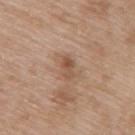Assessment:
The lesion was tiled from a total-body skin photograph and was not biopsied.
Clinical summary:
A female subject aged 73–77. Automated tile analysis of the lesion measured a footprint of about 3.5 mm², an outline eccentricity of about 0.8 (0 = round, 1 = elongated), and a shape-asymmetry score of about 0.35 (0 = symmetric). The analysis additionally found an average lesion color of about L≈52 a*≈19 b*≈30 (CIELAB), about 9 CIELAB-L* units darker than the surrounding skin, and a normalized lesion–skin contrast near 7. The software also gave a border-irregularity rating of about 3.5/10, a color-variation rating of about 2/10, and a peripheral color-asymmetry measure near 0.5. On the upper back. This is a white-light tile. A 15 mm close-up tile from a total-body photography series done for melanoma screening. Approximately 2.5 mm at its widest.